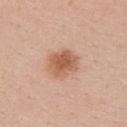workup: catalogued during a skin exam; not biopsied | lighting: white-light illumination | location: the back | patient: female, approximately 25 years of age | acquisition: total-body-photography crop, ~15 mm field of view | automated metrics: border irregularity of about 1.5 on a 0–10 scale, internal color variation of about 3.5 on a 0–10 scale, and peripheral color asymmetry of about 1.